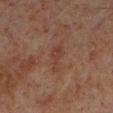Recorded during total-body skin imaging; not selected for excision or biopsy. Automated image analysis of the tile measured a lesion area of about 4.5 mm², an eccentricity of roughly 0.85, and a symmetry-axis asymmetry near 0.5. It also reported a mean CIELAB color near L≈36 a*≈20 b*≈23 and a lesion–skin lightness drop of about 6. The software also gave a border-irregularity index near 5.5/10, internal color variation of about 2 on a 0–10 scale, and a peripheral color-asymmetry measure near 0.5. The analysis additionally found a classifier nevus-likeness of about 0/100. The lesion is on the left lower leg. A male subject, in their 60s. The recorded lesion diameter is about 3.5 mm. A 15 mm close-up tile from a total-body photography series done for melanoma screening. This is a cross-polarized tile.